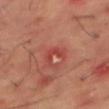Assessment:
Captured during whole-body skin photography for melanoma surveillance; the lesion was not biopsied.
Image and clinical context:
From the right thigh. The lesion-visualizer software estimated a border-irregularity index near 6/10, a color-variation rating of about 0/10, and peripheral color asymmetry of about 0. The software also gave a lesion-detection confidence of about 75/100. The tile uses cross-polarized illumination. A lesion tile, about 15 mm wide, cut from a 3D total-body photograph. The subject is a male roughly 65 years of age. Measured at roughly 2.5 mm in maximum diameter.